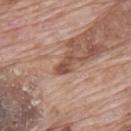The lesion was photographed on a routine skin check and not biopsied; there is no pathology result. Captured under white-light illumination. Cropped from a whole-body photographic skin survey; the tile spans about 15 mm. From the upper back. A male subject, aged 68–72.